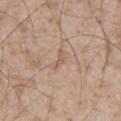On the abdomen.
The lesion-visualizer software estimated a lesion color around L≈58 a*≈17 b*≈29 in CIELAB, a lesion–skin lightness drop of about 7, and a normalized lesion–skin contrast near 5. And it measured a border-irregularity index near 6.5/10, internal color variation of about 0 on a 0–10 scale, and a peripheral color-asymmetry measure near 0.
A 15 mm close-up tile from a total-body photography series done for melanoma screening.
A male subject, in their mid- to late 50s.